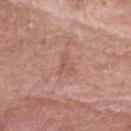notes: total-body-photography surveillance lesion; no biopsy
size: ~3 mm (longest diameter)
anatomic site: the head or neck
image: 15 mm crop, total-body photography
subject: male, roughly 80 years of age